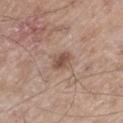notes=total-body-photography surveillance lesion; no biopsy | diameter=~2.5 mm (longest diameter) | image source=~15 mm crop, total-body skin-cancer survey | illumination=white-light | subject=male, about 60 years old | location=the left thigh.